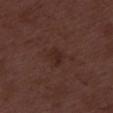Q: Was a biopsy performed?
A: imaged on a skin check; not biopsied
Q: Illumination type?
A: white-light illumination
Q: What kind of image is this?
A: 15 mm crop, total-body photography
Q: What is the lesion's diameter?
A: about 2.5 mm
Q: Who is the patient?
A: male, in their 50s